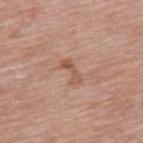biopsy status = imaged on a skin check; not biopsied
imaging modality = total-body-photography crop, ~15 mm field of view
subject = female, aged 58–62
illumination = white-light
location = the upper back